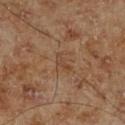image: total-body-photography crop, ~15 mm field of view | location: the leg | subject: male, roughly 70 years of age | TBP lesion metrics: a lesion area of about 2.5 mm² and a symmetry-axis asymmetry near 0.5; a lesion color around L≈42 a*≈18 b*≈30 in CIELAB, about 6 CIELAB-L* units darker than the surrounding skin, and a lesion-to-skin contrast of about 5 (normalized; higher = more distinct); a classifier nevus-likeness of about 0/100 and a lesion-detection confidence of about 100/100 | tile lighting: cross-polarized | size: about 2.5 mm.